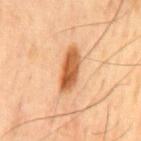| field | value |
|---|---|
| image | ~15 mm crop, total-body skin-cancer survey |
| location | the mid back |
| diameter | about 5.5 mm |
| subject | male, aged around 45 |
| lighting | cross-polarized |